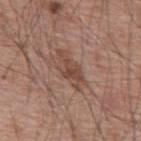Clinical impression: No biopsy was performed on this lesion — it was imaged during a full skin examination and was not determined to be concerning. Image and clinical context: This is a white-light tile. The lesion is on the mid back. A male patient in their mid-50s. A roughly 15 mm field-of-view crop from a total-body skin photograph.